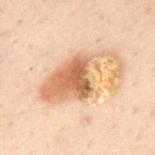follow-up: imaged on a skin check; not biopsied
lesion diameter: ~9 mm (longest diameter)
tile lighting: cross-polarized illumination
subject: male, aged 48–52
acquisition: total-body-photography crop, ~15 mm field of view
TBP lesion metrics: an average lesion color of about L≈55 a*≈17 b*≈31 (CIELAB) and roughly 12 lightness units darker than nearby skin; a nevus-likeness score of about 80/100
anatomic site: the mid back